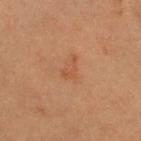  biopsy_status: not biopsied; imaged during a skin examination
  image:
    source: total-body photography crop
    field_of_view_mm: 15
  lighting: cross-polarized
  site: head or neck
  lesion_size:
    long_diameter_mm_approx: 2.5
  patient:
    sex: male
    age_approx: 35